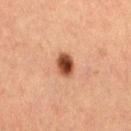Impression: The lesion was tiled from a total-body skin photograph and was not biopsied. Context: Imaged with cross-polarized lighting. A female patient, aged 38 to 42. An algorithmic analysis of the crop reported roughly 17 lightness units darker than nearby skin and a lesion-to-skin contrast of about 13 (normalized; higher = more distinct). And it measured a within-lesion color-variation index near 5/10 and radial color variation of about 1.5. It also reported an automated nevus-likeness rating near 100 out of 100 and lesion-presence confidence of about 100/100. The lesion is on the right thigh. A 15 mm close-up extracted from a 3D total-body photography capture. The lesion's longest dimension is about 2.5 mm.